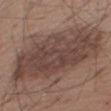Case summary:
- workup — catalogued during a skin exam; not biopsied
- lesion size — ~14 mm (longest diameter)
- patient — male, aged 58 to 62
- body site — the right thigh
- imaging modality — ~15 mm crop, total-body skin-cancer survey
- tile lighting — white-light illumination